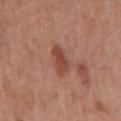The lesion was photographed on a routine skin check and not biopsied; there is no pathology result. This image is a 15 mm lesion crop taken from a total-body photograph. The subject is a male about 70 years old. The lesion is located on the mid back.